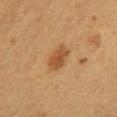Captured during whole-body skin photography for melanoma surveillance; the lesion was not biopsied. The lesion-visualizer software estimated a mean CIELAB color near L≈41 a*≈19 b*≈32. The recorded lesion diameter is about 3.5 mm. A 15 mm close-up extracted from a 3D total-body photography capture. The lesion is on the mid back. Captured under cross-polarized illumination. A female patient, approximately 50 years of age.